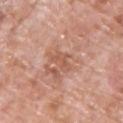Captured during whole-body skin photography for melanoma surveillance; the lesion was not biopsied. Longest diameter approximately 3.5 mm. A male patient about 70 years old. The lesion is on the chest. Automated tile analysis of the lesion measured a footprint of about 4 mm² and a shape-asymmetry score of about 0.45 (0 = symmetric). A region of skin cropped from a whole-body photographic capture, roughly 15 mm wide. Captured under white-light illumination.